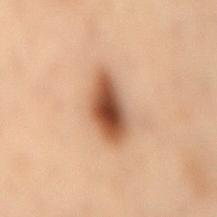The lesion was photographed on a routine skin check and not biopsied; there is no pathology result. On the mid back. A 15 mm close-up extracted from a 3D total-body photography capture. A female subject in their mid- to late 50s.